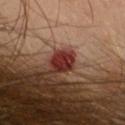This lesion was catalogued during total-body skin photography and was not selected for biopsy.
A lesion tile, about 15 mm wide, cut from a 3D total-body photograph.
Imaged with cross-polarized lighting.
Automated tile analysis of the lesion measured an area of roughly 4.5 mm², an eccentricity of roughly 0.75, and two-axis asymmetry of about 0.25. And it measured peripheral color asymmetry of about 1. It also reported lesion-presence confidence of about 100/100.
The lesion is on the head or neck.
Longest diameter approximately 3 mm.
The patient is a male in their 30s.